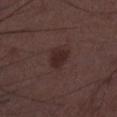Impression:
Part of a total-body skin-imaging series; this lesion was reviewed on a skin check and was not flagged for biopsy.
Context:
The lesion is on the leg. A 15 mm close-up tile from a total-body photography series done for melanoma screening. Captured under white-light illumination. Automated tile analysis of the lesion measured an eccentricity of roughly 0.6 and two-axis asymmetry of about 0.25. The software also gave an average lesion color of about L≈25 a*≈18 b*≈17 (CIELAB), roughly 7 lightness units darker than nearby skin, and a normalized lesion–skin contrast near 8.5. And it measured a border-irregularity index near 2.5/10, internal color variation of about 2 on a 0–10 scale, and peripheral color asymmetry of about 1. The software also gave a nevus-likeness score of about 70/100 and a detector confidence of about 100 out of 100 that the crop contains a lesion. The subject is a male approximately 50 years of age.